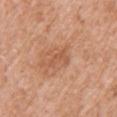workup: catalogued during a skin exam; not biopsied
image source: ~15 mm crop, total-body skin-cancer survey
anatomic site: the right upper arm
size: about 3 mm
patient: female, in their 40s
illumination: white-light
TBP lesion metrics: an eccentricity of roughly 0.9 and a symmetry-axis asymmetry near 0.7; an average lesion color of about L≈55 a*≈24 b*≈34 (CIELAB) and a lesion-to-skin contrast of about 5 (normalized; higher = more distinct); a border-irregularity rating of about 7.5/10 and peripheral color asymmetry of about 0; a nevus-likeness score of about 0/100 and lesion-presence confidence of about 100/100